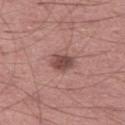| key | value |
|---|---|
| biopsy status | imaged on a skin check; not biopsied |
| patient | male, aged approximately 60 |
| image source | 15 mm crop, total-body photography |
| body site | the left thigh |